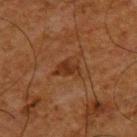Assessment:
No biopsy was performed on this lesion — it was imaged during a full skin examination and was not determined to be concerning.
Image and clinical context:
The patient is a male aged around 65. A lesion tile, about 15 mm wide, cut from a 3D total-body photograph. An algorithmic analysis of the crop reported a footprint of about 5 mm² and an outline eccentricity of about 0.75 (0 = round, 1 = elongated). The analysis additionally found roughly 7 lightness units darker than nearby skin and a normalized lesion–skin contrast near 7. And it measured a border-irregularity rating of about 3.5/10 and a color-variation rating of about 3/10. And it measured a classifier nevus-likeness of about 0/100 and lesion-presence confidence of about 100/100. This is a cross-polarized tile. Located on the upper back.